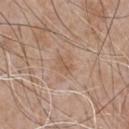  biopsy_status: not biopsied; imaged during a skin examination
  site: chest
  patient:
    sex: male
    age_approx: 65
  image:
    source: total-body photography crop
    field_of_view_mm: 15
  lighting: white-light